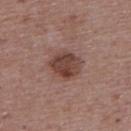{
  "lighting": "white-light",
  "site": "upper back",
  "automated_metrics": {
    "eccentricity": 0.3,
    "shape_asymmetry": 0.15,
    "cielab_L": 42,
    "cielab_a": 20,
    "cielab_b": 23,
    "vs_skin_darker_L": 11.0,
    "vs_skin_contrast_norm": 9.0
  },
  "patient": {
    "sex": "female",
    "age_approx": 65
  },
  "image": {
    "source": "total-body photography crop",
    "field_of_view_mm": 15
  }
}